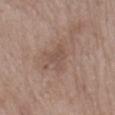biopsy_status: not biopsied; imaged during a skin examination
automated_metrics:
  border_irregularity_0_10: 5.0
  color_variation_0_10: 2.0
  peripheral_color_asymmetry: 0.5
site: mid back
lesion_size:
  long_diameter_mm_approx: 3.5
image:
  source: total-body photography crop
  field_of_view_mm: 15
patient:
  sex: female
  age_approx: 75